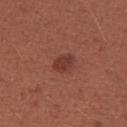biopsy_status: not biopsied; imaged during a skin examination
site: left upper arm
lesion_size:
  long_diameter_mm_approx: 2.5
image:
  source: total-body photography crop
  field_of_view_mm: 15
automated_metrics:
  area_mm2_approx: 4.5
  eccentricity: 0.7
  shape_asymmetry: 0.2
  color_variation_0_10: 1.5
  peripheral_color_asymmetry: 0.5
  nevus_likeness_0_100: 80
patient:
  sex: female
  age_approx: 35